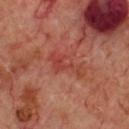Captured during whole-body skin photography for melanoma surveillance; the lesion was not biopsied. A 15 mm close-up tile from a total-body photography series done for melanoma screening. Approximately 4.5 mm at its widest. Captured under cross-polarized illumination. On the chest. The total-body-photography lesion software estimated an area of roughly 6.5 mm² and a symmetry-axis asymmetry near 0.6. The software also gave a border-irregularity index near 8.5/10, a color-variation rating of about 2/10, and peripheral color asymmetry of about 0.5. The subject is a male approximately 70 years of age.